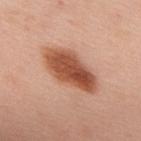Recorded during total-body skin imaging; not selected for excision or biopsy. Measured at roughly 7 mm in maximum diameter. This is a white-light tile. The subject is a male aged 53 to 57. Cropped from a total-body skin-imaging series; the visible field is about 15 mm. From the upper back.